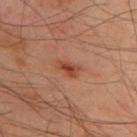Case summary:
• follow-up · catalogued during a skin exam; not biopsied
• anatomic site · the back
• imaging modality · ~15 mm tile from a whole-body skin photo
• patient · male, about 50 years old
• image-analysis metrics · an area of roughly 4 mm², a shape eccentricity near 0.85, and a shape-asymmetry score of about 0.25 (0 = symmetric); a lesion color around L≈45 a*≈27 b*≈32 in CIELAB, a lesion–skin lightness drop of about 9, and a lesion-to-skin contrast of about 7.5 (normalized; higher = more distinct); a border-irregularity rating of about 3/10, a color-variation rating of about 3.5/10, and radial color variation of about 1; a nevus-likeness score of about 80/100 and a lesion-detection confidence of about 100/100